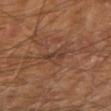automated metrics: a lesion area of about 4 mm² and a shape-asymmetry score of about 0.35 (0 = symmetric); border irregularity of about 4 on a 0–10 scale, a color-variation rating of about 2/10, and radial color variation of about 0.5 | lesion diameter: ≈3 mm | image: ~15 mm crop, total-body skin-cancer survey | location: the right upper arm | subject: male, approximately 70 years of age | illumination: cross-polarized.